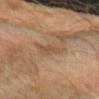biopsy_status: not biopsied; imaged during a skin examination
patient:
  sex: female
  age_approx: 70
site: left forearm
image:
  source: total-body photography crop
  field_of_view_mm: 15
lesion_size:
  long_diameter_mm_approx: 3.0
automated_metrics:
  eccentricity: 0.75
  cielab_L: 44
  cielab_a: 15
  cielab_b: 29
  vs_skin_darker_L: 6.0
  vs_skin_contrast_norm: 5.5
  nevus_likeness_0_100: 0
  lesion_detection_confidence_0_100: 100